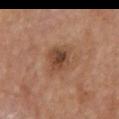The lesion was tiled from a total-body skin photograph and was not biopsied.
The total-body-photography lesion software estimated an eccentricity of roughly 0.6 and a shape-asymmetry score of about 0.2 (0 = symmetric). The analysis additionally found an average lesion color of about L≈43 a*≈19 b*≈29 (CIELAB). And it measured a border-irregularity index near 2.5/10, internal color variation of about 6.5 on a 0–10 scale, and a peripheral color-asymmetry measure near 2. The analysis additionally found a classifier nevus-likeness of about 30/100 and a detector confidence of about 100 out of 100 that the crop contains a lesion.
A male subject, aged 68–72.
The lesion is located on the back.
Measured at roughly 3.5 mm in maximum diameter.
A 15 mm close-up tile from a total-body photography series done for melanoma screening.
This is a cross-polarized tile.